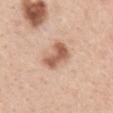Assessment: No biopsy was performed on this lesion — it was imaged during a full skin examination and was not determined to be concerning. Background: Cropped from a total-body skin-imaging series; the visible field is about 15 mm. Automated image analysis of the tile measured a lesion area of about 8.5 mm², a shape eccentricity near 0.85, and a shape-asymmetry score of about 0.25 (0 = symmetric). And it measured a mean CIELAB color near L≈59 a*≈22 b*≈31 and roughly 14 lightness units darker than nearby skin. The software also gave a border-irregularity index near 3/10, a color-variation rating of about 3.5/10, and radial color variation of about 1. Located on the chest. About 4 mm across. The subject is a female aged around 30.